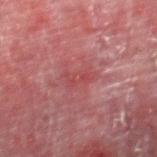Clinical impression:
Recorded during total-body skin imaging; not selected for excision or biopsy.
Background:
Automated image analysis of the tile measured an average lesion color of about L≈41 a*≈29 b*≈21 (CIELAB) and a lesion–skin lightness drop of about 5. And it measured a border-irregularity rating of about 4/10, internal color variation of about 2.5 on a 0–10 scale, and peripheral color asymmetry of about 0.5. A region of skin cropped from a whole-body photographic capture, roughly 15 mm wide. The lesion is on the right thigh. A male subject, in their mid-50s. Captured under cross-polarized illumination.